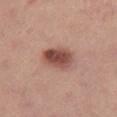{
  "biopsy_status": "not biopsied; imaged during a skin examination"
}